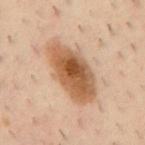biopsy status: no biopsy performed (imaged during a skin exam); size: ≈8 mm; body site: the mid back; patient: male, about 40 years old; acquisition: 15 mm crop, total-body photography.